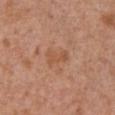Case summary:
• follow-up: imaged on a skin check; not biopsied
• acquisition: ~15 mm tile from a whole-body skin photo
• subject: male, in their mid- to late 60s
• location: the chest